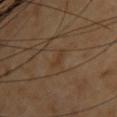Part of a total-body skin-imaging series; this lesion was reviewed on a skin check and was not flagged for biopsy. The lesion is located on the upper back. This image is a 15 mm lesion crop taken from a total-body photograph. A female subject roughly 45 years of age.